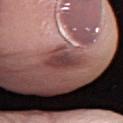Longest diameter approximately 4 mm. Automated tile analysis of the lesion measured a lesion color around L≈41 a*≈23 b*≈20 in CIELAB, roughly 10 lightness units darker than nearby skin, and a lesion-to-skin contrast of about 7.5 (normalized; higher = more distinct). And it measured a nevus-likeness score of about 0/100 and a lesion-detection confidence of about 90/100. Located on the left lower leg. The tile uses white-light illumination. A 15 mm crop from a total-body photograph taken for skin-cancer surveillance. A female subject, approximately 65 years of age.